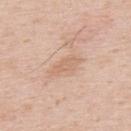Part of a total-body skin-imaging series; this lesion was reviewed on a skin check and was not flagged for biopsy. Automated tile analysis of the lesion measured an eccentricity of roughly 0.9 and a shape-asymmetry score of about 0.25 (0 = symmetric). The software also gave a lesion color around L≈65 a*≈19 b*≈31 in CIELAB, roughly 7 lightness units darker than nearby skin, and a normalized border contrast of about 5. Measured at roughly 3.5 mm in maximum diameter. Imaged with white-light lighting. A close-up tile cropped from a whole-body skin photograph, about 15 mm across. A male patient approximately 50 years of age. The lesion is located on the upper back.